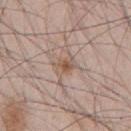Imaged during a routine full-body skin examination; the lesion was not biopsied and no histopathology is available. The lesion-visualizer software estimated a border-irregularity index near 2.5/10, a within-lesion color-variation index near 6/10, and peripheral color asymmetry of about 2. A lesion tile, about 15 mm wide, cut from a 3D total-body photograph. A male patient roughly 45 years of age. Longest diameter approximately 2.5 mm. The lesion is located on the chest.